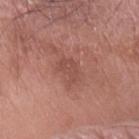Imaged during a routine full-body skin examination; the lesion was not biopsied and no histopathology is available. Imaged with white-light lighting. The patient is a male about 75 years old. A 15 mm close-up tile from a total-body photography series done for melanoma screening. On the left forearm. The total-body-photography lesion software estimated an average lesion color of about L≈49 a*≈25 b*≈25 (CIELAB), roughly 7 lightness units darker than nearby skin, and a lesion-to-skin contrast of about 5 (normalized; higher = more distinct). And it measured border irregularity of about 4 on a 0–10 scale, internal color variation of about 1.5 on a 0–10 scale, and peripheral color asymmetry of about 0.5. It also reported an automated nevus-likeness rating near 0 out of 100 and a detector confidence of about 100 out of 100 that the crop contains a lesion. Longest diameter approximately 3 mm.